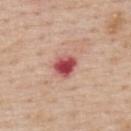Part of a total-body skin-imaging series; this lesion was reviewed on a skin check and was not flagged for biopsy. On the back. A region of skin cropped from a whole-body photographic capture, roughly 15 mm wide. A male subject, about 60 years old.